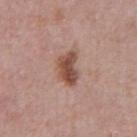Captured during whole-body skin photography for melanoma surveillance; the lesion was not biopsied.
A roughly 15 mm field-of-view crop from a total-body skin photograph.
On the front of the torso.
The patient is a male in their 70s.